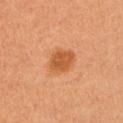Notes:
* follow-up: no biopsy performed (imaged during a skin exam)
* image source: ~15 mm crop, total-body skin-cancer survey
* lesion size: ~3.5 mm (longest diameter)
* subject: female, aged around 50
* image-analysis metrics: an area of roughly 8 mm², a shape eccentricity near 0.5, and a shape-asymmetry score of about 0.2 (0 = symmetric); a mean CIELAB color near L≈56 a*≈28 b*≈43, a lesion–skin lightness drop of about 11, and a normalized lesion–skin contrast near 7.5; a nevus-likeness score of about 95/100 and lesion-presence confidence of about 100/100
* illumination: cross-polarized illumination